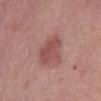biopsy status: no biopsy performed (imaged during a skin exam); imaging modality: ~15 mm crop, total-body skin-cancer survey; image-analysis metrics: a footprint of about 11 mm² and a shape eccentricity near 0.75; illumination: white-light; location: the front of the torso; patient: female, roughly 65 years of age; lesion diameter: ~4.5 mm (longest diameter).